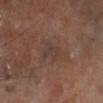Case summary:
• biopsy status · no biopsy performed (imaged during a skin exam)
• image-analysis metrics · an eccentricity of roughly 0.75; an automated nevus-likeness rating near 0 out of 100
• tile lighting · cross-polarized
• image source · ~15 mm crop, total-body skin-cancer survey
• lesion size · about 2.5 mm
• anatomic site · the right lower leg